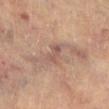Clinical impression: Captured during whole-body skin photography for melanoma surveillance; the lesion was not biopsied. Image and clinical context: Located on the left lower leg. The patient is a female aged around 80. A region of skin cropped from a whole-body photographic capture, roughly 15 mm wide.